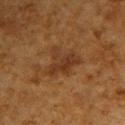follow-up: total-body-photography surveillance lesion; no biopsy | location: the upper back | image: total-body-photography crop, ~15 mm field of view | patient: male, aged 58–62 | tile lighting: cross-polarized illumination | automated lesion analysis: a footprint of about 10 mm², a shape eccentricity near 0.6, and a symmetry-axis asymmetry near 0.4; an average lesion color of about L≈29 a*≈18 b*≈28 (CIELAB) and a lesion-to-skin contrast of about 6.5 (normalized; higher = more distinct); border irregularity of about 5.5 on a 0–10 scale, internal color variation of about 3 on a 0–10 scale, and a peripheral color-asymmetry measure near 1; a classifier nevus-likeness of about 5/100 and a lesion-detection confidence of about 100/100.